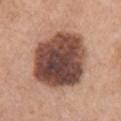workup: total-body-photography surveillance lesion; no biopsy
image: 15 mm crop, total-body photography
lesion diameter: ≈7.5 mm
image-analysis metrics: an average lesion color of about L≈46 a*≈20 b*≈25 (CIELAB), a lesion–skin lightness drop of about 21, and a normalized border contrast of about 14
subject: female, approximately 60 years of age
location: the right upper arm
illumination: white-light illumination